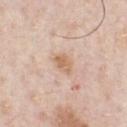Clinical impression:
The lesion was tiled from a total-body skin photograph and was not biopsied.
Image and clinical context:
The lesion's longest dimension is about 2.5 mm. A male patient, aged 58 to 62. Imaged with white-light lighting. The lesion is located on the chest. This image is a 15 mm lesion crop taken from a total-body photograph. Automated image analysis of the tile measured a footprint of about 4 mm² and two-axis asymmetry of about 0.3. The analysis additionally found roughly 9 lightness units darker than nearby skin and a lesion-to-skin contrast of about 7 (normalized; higher = more distinct).